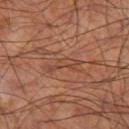Clinical impression: Part of a total-body skin-imaging series; this lesion was reviewed on a skin check and was not flagged for biopsy. Background: The lesion-visualizer software estimated a border-irregularity rating of about 6.5/10. The analysis additionally found an automated nevus-likeness rating near 0 out of 100 and a detector confidence of about 70 out of 100 that the crop contains a lesion. The tile uses cross-polarized illumination. The recorded lesion diameter is about 4 mm. The lesion is on the left thigh. A 15 mm close-up tile from a total-body photography series done for melanoma screening. The patient is a male in their 60s.